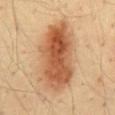  biopsy_status: not biopsied; imaged during a skin examination
  site: mid back
  image:
    source: total-body photography crop
    field_of_view_mm: 15
  lighting: cross-polarized
  patient:
    sex: male
    age_approx: 35